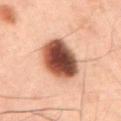<lesion>
  <biopsy_status>not biopsied; imaged during a skin examination</biopsy_status>
  <site>abdomen</site>
  <lighting>cross-polarized</lighting>
  <image>
    <source>total-body photography crop</source>
    <field_of_view_mm>15</field_of_view_mm>
  </image>
  <patient>
    <sex>male</sex>
    <age_approx>60</age_approx>
  </patient>
  <lesion_size>
    <long_diameter_mm_approx>5.5</long_diameter_mm_approx>
  </lesion_size>
</lesion>